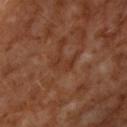Clinical summary: The total-body-photography lesion software estimated an area of roughly 4 mm², an outline eccentricity of about 0.8 (0 = round, 1 = elongated), and a symmetry-axis asymmetry near 0.3. And it measured roughly 5 lightness units darker than nearby skin and a normalized lesion–skin contrast near 5. Imaged with cross-polarized lighting. The lesion's longest dimension is about 2.5 mm. This image is a 15 mm lesion crop taken from a total-body photograph. A male patient aged 58–62. From the right upper arm.